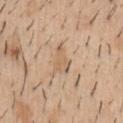No biopsy was performed on this lesion — it was imaged during a full skin examination and was not determined to be concerning. Cropped from a total-body skin-imaging series; the visible field is about 15 mm. Automated tile analysis of the lesion measured a footprint of about 5.5 mm² and an eccentricity of roughly 0.85. It also reported an average lesion color of about L≈62 a*≈16 b*≈33 (CIELAB), a lesion–skin lightness drop of about 8, and a lesion-to-skin contrast of about 5 (normalized; higher = more distinct). And it measured a classifier nevus-likeness of about 0/100. A male patient aged 38 to 42. The lesion is located on the abdomen. About 3.5 mm across. Captured under white-light illumination.